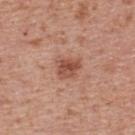Captured during whole-body skin photography for melanoma surveillance; the lesion was not biopsied. From the upper back. Longest diameter approximately 3 mm. A lesion tile, about 15 mm wide, cut from a 3D total-body photograph. A male patient aged 68 to 72.